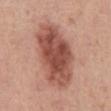{
  "biopsy_status": "not biopsied; imaged during a skin examination",
  "image": {
    "source": "total-body photography crop",
    "field_of_view_mm": 15
  },
  "lighting": "white-light",
  "lesion_size": {
    "long_diameter_mm_approx": 9.5
  },
  "automated_metrics": {
    "border_irregularity_0_10": 2.5,
    "color_variation_0_10": 6.0,
    "peripheral_color_asymmetry": 2.0,
    "nevus_likeness_0_100": 80,
    "lesion_detection_confidence_0_100": 100
  },
  "site": "abdomen",
  "patient": {
    "sex": "male",
    "age_approx": 50
  }
}